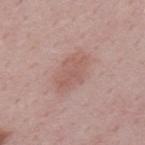biopsy_status: not biopsied; imaged during a skin examination
site: upper back
patient:
  sex: male
  age_approx: 50
lesion_size:
  long_diameter_mm_approx: 5.5
automated_metrics:
  eccentricity: 0.8
  shape_asymmetry: 0.15
  border_irregularity_0_10: 2.0
  color_variation_0_10: 2.5
  peripheral_color_asymmetry: 1.0
  lesion_detection_confidence_0_100: 100
image:
  source: total-body photography crop
  field_of_view_mm: 15